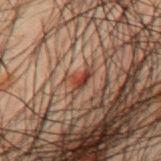tile lighting = cross-polarized illumination | anatomic site = the mid back | imaging modality = total-body-photography crop, ~15 mm field of view | patient = male, roughly 50 years of age | diameter = ≈3 mm.